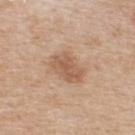Part of a total-body skin-imaging series; this lesion was reviewed on a skin check and was not flagged for biopsy. On the upper back. Approximately 4 mm at its widest. The tile uses white-light illumination. The patient is a male aged 58–62. Automated image analysis of the tile measured a footprint of about 9.5 mm² and an eccentricity of roughly 0.75. It also reported a within-lesion color-variation index near 2.5/10 and a peripheral color-asymmetry measure near 1. And it measured a classifier nevus-likeness of about 5/100 and lesion-presence confidence of about 100/100. A 15 mm close-up tile from a total-body photography series done for melanoma screening.